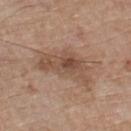patient: male, aged 73 to 77
acquisition: 15 mm crop, total-body photography
TBP lesion metrics: a nevus-likeness score of about 15/100 and a detector confidence of about 100 out of 100 that the crop contains a lesion
location: the chest
lighting: white-light illumination
lesion size: ~7 mm (longest diameter)